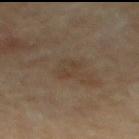Clinical summary:
The tile uses cross-polarized illumination. The lesion is located on the back. The patient is a male aged around 85 or older. About 3 mm across. This image is a 15 mm lesion crop taken from a total-body photograph.